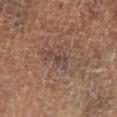notes=total-body-photography surveillance lesion; no biopsy | anatomic site=the left lower leg | illumination=cross-polarized | image=15 mm crop, total-body photography | size=≈3 mm | automated metrics=a lesion area of about 3 mm², an eccentricity of roughly 0.9, and two-axis asymmetry of about 0.5; roughly 6 lightness units darker than nearby skin and a normalized lesion–skin contrast near 6.5; a color-variation rating of about 0/10 and a peripheral color-asymmetry measure near 0 | subject=male, aged 63–67.